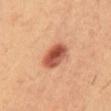Q: Was this lesion biopsied?
A: imaged on a skin check; not biopsied
Q: What is the imaging modality?
A: ~15 mm tile from a whole-body skin photo
Q: What is the anatomic site?
A: the abdomen
Q: What lighting was used for the tile?
A: cross-polarized
Q: How large is the lesion?
A: ≈4 mm
Q: Who is the patient?
A: male, roughly 50 years of age